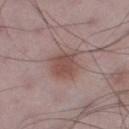workup: imaged on a skin check; not biopsied
acquisition: 15 mm crop, total-body photography
anatomic site: the right thigh
subject: male, in their 50s